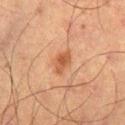biopsy status — imaged on a skin check; not biopsied
anatomic site — the left thigh
diameter — ≈3 mm
image source — 15 mm crop, total-body photography
patient — male, approximately 65 years of age
TBP lesion metrics — a lesion color around L≈45 a*≈21 b*≈32 in CIELAB, a lesion–skin lightness drop of about 9, and a normalized border contrast of about 7